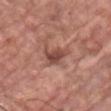A close-up tile cropped from a whole-body skin photograph, about 15 mm across.
The subject is a male aged approximately 80.
From the chest.